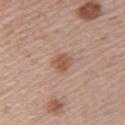Assessment:
Part of a total-body skin-imaging series; this lesion was reviewed on a skin check and was not flagged for biopsy.
Background:
A female subject about 50 years old. The lesion's longest dimension is about 3 mm. The lesion is on the arm. Automated tile analysis of the lesion measured a lesion color around L≈55 a*≈20 b*≈29 in CIELAB, a lesion–skin lightness drop of about 9, and a normalized border contrast of about 7. The software also gave a nevus-likeness score of about 65/100. The tile uses white-light illumination. A 15 mm close-up tile from a total-body photography series done for melanoma screening.